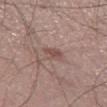Impression: The lesion was tiled from a total-body skin photograph and was not biopsied. Background: Measured at roughly 2.5 mm in maximum diameter. A 15 mm close-up extracted from a 3D total-body photography capture. This is a white-light tile. A male patient, roughly 55 years of age. Located on the left thigh.